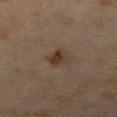patient=female, in their mid- to late 50s | imaging modality=15 mm crop, total-body photography | automated metrics=a color-variation rating of about 4/10 and peripheral color asymmetry of about 1.5; an automated nevus-likeness rating near 85 out of 100 and a detector confidence of about 100 out of 100 that the crop contains a lesion | anatomic site=the right lower leg | lesion size=about 3 mm | lighting=cross-polarized.